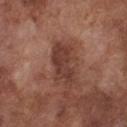Findings:
* notes · no biopsy performed (imaged during a skin exam)
* body site · the chest
* subject · male, aged around 75
* image-analysis metrics · a border-irregularity index near 4.5/10 and internal color variation of about 2.5 on a 0–10 scale; a classifier nevus-likeness of about 0/100 and a lesion-detection confidence of about 100/100
* lighting · white-light
* imaging modality · total-body-photography crop, ~15 mm field of view
* lesion diameter · about 5.5 mm